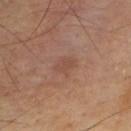The lesion was tiled from a total-body skin photograph and was not biopsied. Cropped from a whole-body photographic skin survey; the tile spans about 15 mm. Located on the upper back. The recorded lesion diameter is about 2.5 mm. A male patient aged approximately 45.